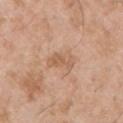{
  "biopsy_status": "not biopsied; imaged during a skin examination",
  "image": {
    "source": "total-body photography crop",
    "field_of_view_mm": 15
  },
  "site": "upper back",
  "lighting": "white-light",
  "lesion_size": {
    "long_diameter_mm_approx": 3.5
  },
  "patient": {
    "sex": "male",
    "age_approx": 55
  }
}